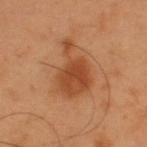Part of a total-body skin-imaging series; this lesion was reviewed on a skin check and was not flagged for biopsy. On the upper back. The patient is a male in their mid-50s. This image is a 15 mm lesion crop taken from a total-body photograph.